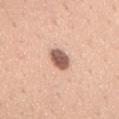Findings:
- follow-up — total-body-photography surveillance lesion; no biopsy
- lesion size — about 3.5 mm
- acquisition — 15 mm crop, total-body photography
- patient — male, roughly 30 years of age
- site — the front of the torso